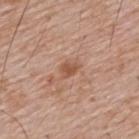The lesion was photographed on a routine skin check and not biopsied; there is no pathology result.
Captured under white-light illumination.
The patient is a male aged around 65.
From the upper back.
A 15 mm close-up tile from a total-body photography series done for melanoma screening.
Longest diameter approximately 2.5 mm.